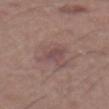| feature | finding |
|---|---|
| workup | total-body-photography surveillance lesion; no biopsy |
| image | 15 mm crop, total-body photography |
| body site | the abdomen |
| diameter | ~3.5 mm (longest diameter) |
| image-analysis metrics | an area of roughly 5.5 mm², an eccentricity of roughly 0.8, and two-axis asymmetry of about 0.3; a mean CIELAB color near L≈47 a*≈19 b*≈18 and a lesion–skin lightness drop of about 7; an automated nevus-likeness rating near 5 out of 100 and a detector confidence of about 100 out of 100 that the crop contains a lesion |
| patient | male, approximately 55 years of age |